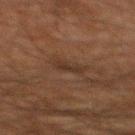Assessment: Part of a total-body skin-imaging series; this lesion was reviewed on a skin check and was not flagged for biopsy. Clinical summary: This is a cross-polarized tile. The lesion is located on the mid back. A lesion tile, about 15 mm wide, cut from a 3D total-body photograph. Longest diameter approximately 2.5 mm. The patient is a male roughly 60 years of age.